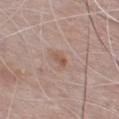Captured during whole-body skin photography for melanoma surveillance; the lesion was not biopsied.
About 2.5 mm across.
From the chest.
Imaged with white-light lighting.
A male patient, aged 73 to 77.
The total-body-photography lesion software estimated border irregularity of about 2.5 on a 0–10 scale and a color-variation rating of about 3.5/10. And it measured an automated nevus-likeness rating near 0 out of 100 and lesion-presence confidence of about 100/100.
A close-up tile cropped from a whole-body skin photograph, about 15 mm across.